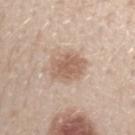{
  "biopsy_status": "not biopsied; imaged during a skin examination",
  "site": "left forearm",
  "patient": {
    "sex": "male",
    "age_approx": 30
  },
  "image": {
    "source": "total-body photography crop",
    "field_of_view_mm": 15
  }
}